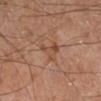Clinical impression:
Recorded during total-body skin imaging; not selected for excision or biopsy.
Image and clinical context:
A male subject, in their mid- to late 60s. From the right lower leg. Imaged with cross-polarized lighting. A 15 mm close-up tile from a total-body photography series done for melanoma screening.